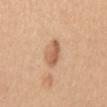biopsy_status: not biopsied; imaged during a skin examination
lighting: white-light
patient:
  sex: female
  age_approx: 55
automated_metrics:
  area_mm2_approx: 6.5
  eccentricity: 0.85
  shape_asymmetry: 0.2
  vs_skin_darker_L: 11.0
  vs_skin_contrast_norm: 7.5
  border_irregularity_0_10: 2.5
  color_variation_0_10: 4.0
  peripheral_color_asymmetry: 1.5
image:
  source: total-body photography crop
  field_of_view_mm: 15
lesion_size:
  long_diameter_mm_approx: 4.0
site: chest